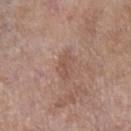<lesion>
<biopsy_status>not biopsied; imaged during a skin examination</biopsy_status>
<lesion_size>
  <long_diameter_mm_approx>3.5</long_diameter_mm_approx>
</lesion_size>
<image>
  <source>total-body photography crop</source>
  <field_of_view_mm>15</field_of_view_mm>
</image>
<patient>
  <sex>female</sex>
  <age_approx>85+</age_approx>
</patient>
<automated_metrics>
  <vs_skin_darker_L>7.0</vs_skin_darker_L>
  <vs_skin_contrast_norm>5.0</vs_skin_contrast_norm>
  <border_irregularity_0_10>3.0</border_irregularity_0_10>
  <color_variation_0_10>2.0</color_variation_0_10>
</automated_metrics>
<lighting>white-light</lighting>
<site>left lower leg</site>
</lesion>